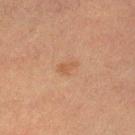{"biopsy_status": "not biopsied; imaged during a skin examination", "lesion_size": {"long_diameter_mm_approx": 2.5}, "image": {"source": "total-body photography crop", "field_of_view_mm": 15}, "lighting": "cross-polarized", "patient": {"sex": "female", "age_approx": 55}, "site": "leg"}